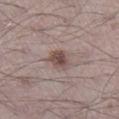Q: Was a biopsy performed?
A: no biopsy performed (imaged during a skin exam)
Q: What is the lesion's diameter?
A: ≈3 mm
Q: Who is the patient?
A: male, in their 70s
Q: How was this image acquired?
A: ~15 mm crop, total-body skin-cancer survey
Q: Automated lesion metrics?
A: a lesion area of about 5 mm² and a shape-asymmetry score of about 0.25 (0 = symmetric)
Q: What is the anatomic site?
A: the right lower leg